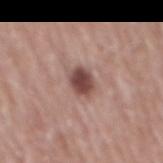workup = no biopsy performed (imaged during a skin exam); subject = male, approximately 70 years of age; body site = the mid back; image source = ~15 mm tile from a whole-body skin photo; lesion diameter = ≈3 mm.